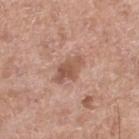Imaged during a routine full-body skin examination; the lesion was not biopsied and no histopathology is available.
From the right lower leg.
Longest diameter approximately 4 mm.
The subject is a male approximately 60 years of age.
Imaged with white-light lighting.
Cropped from a total-body skin-imaging series; the visible field is about 15 mm.